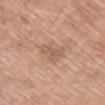follow-up: imaged on a skin check; not biopsied | subject: female, about 65 years old | image-analysis metrics: an average lesion color of about L≈58 a*≈20 b*≈29 (CIELAB) and a lesion-to-skin contrast of about 5 (normalized; higher = more distinct) | image source: total-body-photography crop, ~15 mm field of view | lighting: white-light | body site: the chest.